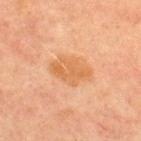Context: From the upper back. This image is a 15 mm lesion crop taken from a total-body photograph. The tile uses cross-polarized illumination. A male patient, aged approximately 60.